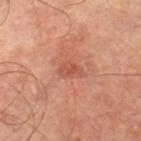<case>
  <biopsy_status>not biopsied; imaged during a skin examination</biopsy_status>
  <lesion_size>
    <long_diameter_mm_approx>3.0</long_diameter_mm_approx>
  </lesion_size>
  <lighting>cross-polarized</lighting>
  <image>
    <source>total-body photography crop</source>
    <field_of_view_mm>15</field_of_view_mm>
  </image>
  <patient>
    <sex>male</sex>
    <age_approx>65</age_approx>
  </patient>
  <automated_metrics>
    <cielab_L>53</cielab_L>
    <cielab_a>29</cielab_a>
    <cielab_b>32</cielab_b>
    <vs_skin_darker_L>8.0</vs_skin_darker_L>
    <vs_skin_contrast_norm>5.5</vs_skin_contrast_norm>
  </automated_metrics>
  <site>right lower leg</site>
</case>